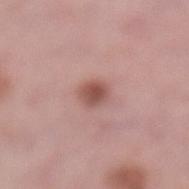Clinical impression: No biopsy was performed on this lesion — it was imaged during a full skin examination and was not determined to be concerning. Acquisition and patient details: This is a white-light tile. About 2.5 mm across. A 15 mm close-up tile from a total-body photography series done for melanoma screening. A female subject, in their mid-60s. On the left lower leg.